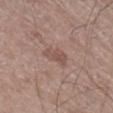Q: Was a biopsy performed?
A: catalogued during a skin exam; not biopsied
Q: Patient demographics?
A: male, roughly 65 years of age
Q: Where on the body is the lesion?
A: the right lower leg
Q: What kind of image is this?
A: total-body-photography crop, ~15 mm field of view
Q: What lighting was used for the tile?
A: white-light